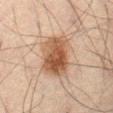No biopsy was performed on this lesion — it was imaged during a full skin examination and was not determined to be concerning. A lesion tile, about 15 mm wide, cut from a 3D total-body photograph. A male patient, aged around 70. This is a cross-polarized tile. Measured at roughly 5.5 mm in maximum diameter. The lesion-visualizer software estimated a border-irregularity rating of about 2.5/10, internal color variation of about 6 on a 0–10 scale, and peripheral color asymmetry of about 2. The lesion is on the front of the torso.